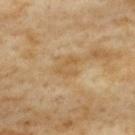Impression:
Recorded during total-body skin imaging; not selected for excision or biopsy.
Clinical summary:
A female subject, aged 58 to 62. Automated image analysis of the tile measured a lesion area of about 4.5 mm², a shape eccentricity near 0.75, and two-axis asymmetry of about 0.45. The software also gave an average lesion color of about L≈52 a*≈14 b*≈36 (CIELAB), about 6 CIELAB-L* units darker than the surrounding skin, and a normalized lesion–skin contrast near 5. It also reported border irregularity of about 5 on a 0–10 scale and a peripheral color-asymmetry measure near 0. On the upper back. The lesion's longest dimension is about 3 mm. A roughly 15 mm field-of-view crop from a total-body skin photograph. Imaged with cross-polarized lighting.